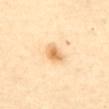notes = catalogued during a skin exam; not biopsied
location = the abdomen
diameter = ≈2.5 mm
imaging modality = ~15 mm crop, total-body skin-cancer survey
patient = female, in their mid-50s